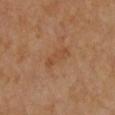The lesion was photographed on a routine skin check and not biopsied; there is no pathology result. A female subject aged 43–47. This is a cross-polarized tile. The lesion is on the chest. Measured at roughly 4 mm in maximum diameter. A roughly 15 mm field-of-view crop from a total-body skin photograph.